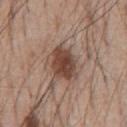The lesion was photographed on a routine skin check and not biopsied; there is no pathology result. A close-up tile cropped from a whole-body skin photograph, about 15 mm across. The lesion is on the chest. The lesion's longest dimension is about 4.5 mm. The patient is a male aged approximately 55. Imaged with white-light lighting.